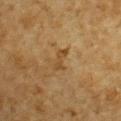A male subject approximately 85 years of age or older. A close-up tile cropped from a whole-body skin photograph, about 15 mm across. Located on the chest.